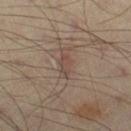• notes · no biopsy performed (imaged during a skin exam)
• image · 15 mm crop, total-body photography
• image-analysis metrics · a footprint of about 3.5 mm², an eccentricity of roughly 0.9, and a shape-asymmetry score of about 0.35 (0 = symmetric); an average lesion color of about L≈46 a*≈16 b*≈23 (CIELAB), roughly 7 lightness units darker than nearby skin, and a lesion-to-skin contrast of about 5.5 (normalized; higher = more distinct); a lesion-detection confidence of about 95/100
• illumination · cross-polarized
• subject · male, approximately 55 years of age
• site · the leg
• lesion diameter · about 3 mm